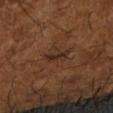notes: catalogued during a skin exam; not biopsied | image-analysis metrics: an area of roughly 3.5 mm² and a shape-asymmetry score of about 0.35 (0 = symmetric) | lighting: cross-polarized illumination | patient: male, approximately 65 years of age | location: the right upper arm | image source: total-body-photography crop, ~15 mm field of view | size: ~3 mm (longest diameter).